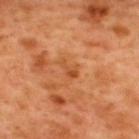Captured during whole-body skin photography for melanoma surveillance; the lesion was not biopsied.
A male subject, roughly 50 years of age.
This is a cross-polarized tile.
The lesion is on the back.
A close-up tile cropped from a whole-body skin photograph, about 15 mm across.